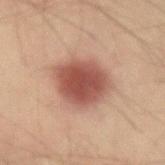notes = total-body-photography surveillance lesion; no biopsy
imaging modality = 15 mm crop, total-body photography
subject = male, approximately 40 years of age
body site = the arm